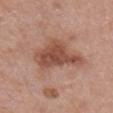<case>
  <biopsy_status>not biopsied; imaged during a skin examination</biopsy_status>
  <site>chest</site>
  <patient>
    <sex>female</sex>
    <age_approx>65</age_approx>
  </patient>
  <image>
    <source>total-body photography crop</source>
    <field_of_view_mm>15</field_of_view_mm>
  </image>
  <lighting>white-light</lighting>
  <automated_metrics>
    <cielab_L>50</cielab_L>
    <cielab_a>23</cielab_a>
    <cielab_b>28</cielab_b>
    <vs_skin_darker_L>12.0</vs_skin_darker_L>
    <border_irregularity_0_10>5.0</border_irregularity_0_10>
    <color_variation_0_10>4.0</color_variation_0_10>
    <nevus_likeness_0_100>25</nevus_likeness_0_100>
    <lesion_detection_confidence_0_100>100</lesion_detection_confidence_0_100>
  </automated_metrics>
  <lesion_size>
    <long_diameter_mm_approx>6.5</long_diameter_mm_approx>
  </lesion_size>
</case>